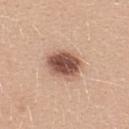workup: catalogued during a skin exam; not biopsied
location: the upper back
lesion size: about 4.5 mm
image: 15 mm crop, total-body photography
image-analysis metrics: a footprint of about 11 mm², a shape eccentricity near 0.65, and a symmetry-axis asymmetry near 0.15; a classifier nevus-likeness of about 95/100 and a lesion-detection confidence of about 100/100
illumination: white-light illumination
subject: female, aged around 25